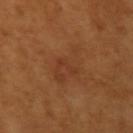A roughly 15 mm field-of-view crop from a total-body skin photograph.
The subject is a female roughly 55 years of age.
Captured under cross-polarized illumination.
Located on the left upper arm.
Approximately 3 mm at its widest.
The lesion-visualizer software estimated a footprint of about 2.5 mm². And it measured radial color variation of about 0. And it measured a classifier nevus-likeness of about 0/100 and lesion-presence confidence of about 100/100.